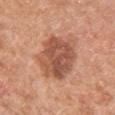workup: total-body-photography surveillance lesion; no biopsy
body site: the chest
subject: male, about 30 years old
image: 15 mm crop, total-body photography
automated lesion analysis: a mean CIELAB color near L≈54 a*≈24 b*≈32 and a lesion-to-skin contrast of about 8.5 (normalized; higher = more distinct); peripheral color asymmetry of about 2; an automated nevus-likeness rating near 30 out of 100 and a lesion-detection confidence of about 100/100
tile lighting: white-light illumination
diameter: ≈7 mm